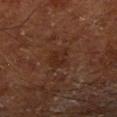biopsy status: imaged on a skin check; not biopsied
patient: male, aged 63–67
automated lesion analysis: a lesion area of about 5.5 mm², a shape eccentricity near 0.45, and two-axis asymmetry of about 0.3; a border-irregularity index near 3.5/10, internal color variation of about 2.5 on a 0–10 scale, and peripheral color asymmetry of about 1; a nevus-likeness score of about 10/100
acquisition: total-body-photography crop, ~15 mm field of view
lighting: cross-polarized
body site: the leg